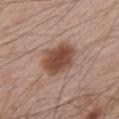<lesion>
<biopsy_status>not biopsied; imaged during a skin examination</biopsy_status>
<image>
  <source>total-body photography crop</source>
  <field_of_view_mm>15</field_of_view_mm>
</image>
<patient>
  <sex>male</sex>
  <age_approx>65</age_approx>
</patient>
<lighting>white-light</lighting>
<automated_metrics>
  <area_mm2_approx>14.0</area_mm2_approx>
  <eccentricity>0.65</eccentricity>
  <shape_asymmetry>0.15</shape_asymmetry>
  <vs_skin_darker_L>14.0</vs_skin_darker_L>
  <vs_skin_contrast_norm>10.0</vs_skin_contrast_norm>
</automated_metrics>
<site>chest</site>
</lesion>